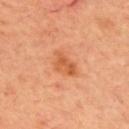Part of a total-body skin-imaging series; this lesion was reviewed on a skin check and was not flagged for biopsy.
A close-up tile cropped from a whole-body skin photograph, about 15 mm across.
On the upper back.
A male subject roughly 70 years of age.
An algorithmic analysis of the crop reported a lesion color around L≈56 a*≈28 b*≈40 in CIELAB, about 9 CIELAB-L* units darker than the surrounding skin, and a normalized border contrast of about 6.5. And it measured a border-irregularity rating of about 4/10, a color-variation rating of about 3/10, and a peripheral color-asymmetry measure near 1.